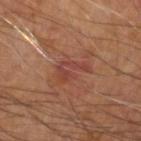Q: Was a biopsy performed?
A: imaged on a skin check; not biopsied
Q: Lesion location?
A: the right forearm
Q: Illumination type?
A: cross-polarized illumination
Q: What kind of image is this?
A: ~15 mm tile from a whole-body skin photo
Q: What is the lesion's diameter?
A: about 3.5 mm
Q: Patient demographics?
A: male, about 70 years old